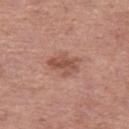Part of a total-body skin-imaging series; this lesion was reviewed on a skin check and was not flagged for biopsy. A female subject aged approximately 40. A lesion tile, about 15 mm wide, cut from a 3D total-body photograph. From the left thigh. Imaged with white-light lighting. The lesion's longest dimension is about 4 mm.